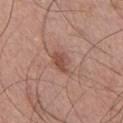Q: Was this lesion biopsied?
A: imaged on a skin check; not biopsied
Q: What is the lesion's diameter?
A: ~3 mm (longest diameter)
Q: Where on the body is the lesion?
A: the chest
Q: Patient demographics?
A: male, aged 28–32
Q: What kind of image is this?
A: ~15 mm tile from a whole-body skin photo